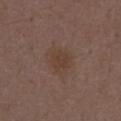No biopsy was performed on this lesion — it was imaged during a full skin examination and was not determined to be concerning. This is a white-light tile. A male patient in their 50s. The recorded lesion diameter is about 3 mm. An algorithmic analysis of the crop reported a lesion–skin lightness drop of about 6. It also reported a border-irregularity rating of about 2/10. The analysis additionally found a nevus-likeness score of about 5/100. A region of skin cropped from a whole-body photographic capture, roughly 15 mm wide. The lesion is located on the front of the torso.